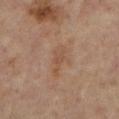Q: Was this lesion biopsied?
A: total-body-photography surveillance lesion; no biopsy
Q: What is the lesion's diameter?
A: ≈4.5 mm
Q: What did automated image analysis measure?
A: a footprint of about 7.5 mm², an eccentricity of roughly 0.9, and a shape-asymmetry score of about 0.4 (0 = symmetric); an automated nevus-likeness rating near 0 out of 100
Q: Lesion location?
A: the right lower leg
Q: What are the patient's age and sex?
A: female, about 75 years old
Q: How was this image acquired?
A: ~15 mm crop, total-body skin-cancer survey